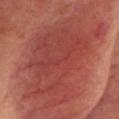No biopsy was performed on this lesion — it was imaged during a full skin examination and was not determined to be concerning. On the head or neck. A 15 mm crop from a total-body photograph taken for skin-cancer surveillance. The subject is female. The lesion's longest dimension is about 14.5 mm.